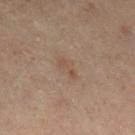biopsy_status: not biopsied; imaged during a skin examination
patient:
  sex: male
  age_approx: 55
lighting: cross-polarized
image:
  source: total-body photography crop
  field_of_view_mm: 15
site: right lower leg
lesion_size:
  long_diameter_mm_approx: 3.0